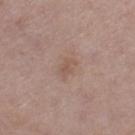No biopsy was performed on this lesion — it was imaged during a full skin examination and was not determined to be concerning.
Measured at roughly 2.5 mm in maximum diameter.
From the left thigh.
A male subject, aged 68–72.
The tile uses white-light illumination.
A 15 mm close-up tile from a total-body photography series done for melanoma screening.
The lesion-visualizer software estimated an average lesion color of about L≈54 a*≈17 b*≈25 (CIELAB), a lesion–skin lightness drop of about 6, and a normalized lesion–skin contrast near 4.5. It also reported a border-irregularity index near 3/10.